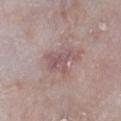follow-up: total-body-photography surveillance lesion; no biopsy
lesion diameter: ~4.5 mm (longest diameter)
body site: the right lower leg
illumination: white-light illumination
TBP lesion metrics: an outline eccentricity of about 0.7 (0 = round, 1 = elongated); a mean CIELAB color near L≈54 a*≈19 b*≈18, a lesion–skin lightness drop of about 8, and a normalized lesion–skin contrast near 6; a nevus-likeness score of about 0/100 and a detector confidence of about 90 out of 100 that the crop contains a lesion
subject: male, aged 63 to 67
image: ~15 mm tile from a whole-body skin photo